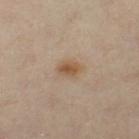Case summary:
– biopsy status · catalogued during a skin exam; not biopsied
– patient · female, aged 38–42
– image · total-body-photography crop, ~15 mm field of view
– location · the right leg